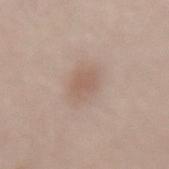Q: Was this lesion biopsied?
A: no biopsy performed (imaged during a skin exam)
Q: What is the anatomic site?
A: the lower back
Q: Who is the patient?
A: female, in their mid-60s
Q: What lighting was used for the tile?
A: white-light illumination
Q: What is the imaging modality?
A: 15 mm crop, total-body photography